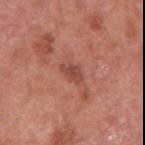On the right upper arm.
A male subject aged approximately 70.
The lesion-visualizer software estimated a lesion area of about 3 mm² and a shape-asymmetry score of about 0.4 (0 = symmetric). And it measured a normalized border contrast of about 7. And it measured a nevus-likeness score of about 5/100 and lesion-presence confidence of about 100/100.
The recorded lesion diameter is about 3 mm.
The tile uses white-light illumination.
A close-up tile cropped from a whole-body skin photograph, about 15 mm across.